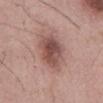Clinical impression: Recorded during total-body skin imaging; not selected for excision or biopsy. Context: A male patient aged 53 to 57. The tile uses white-light illumination. About 6.5 mm across. Automated tile analysis of the lesion measured a footprint of about 15 mm², an outline eccentricity of about 0.9 (0 = round, 1 = elongated), and two-axis asymmetry of about 0.2. The analysis additionally found a mean CIELAB color near L≈51 a*≈21 b*≈23, about 13 CIELAB-L* units darker than the surrounding skin, and a normalized border contrast of about 9. And it measured border irregularity of about 3 on a 0–10 scale, a within-lesion color-variation index near 5/10, and a peripheral color-asymmetry measure near 1.5. It also reported a nevus-likeness score of about 75/100 and lesion-presence confidence of about 100/100. A 15 mm close-up extracted from a 3D total-body photography capture. On the mid back.